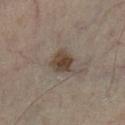* follow-up: no biopsy performed (imaged during a skin exam)
* image: total-body-photography crop, ~15 mm field of view
* location: the left lower leg
* lighting: cross-polarized illumination
* diameter: ≈3 mm
* patient: male, aged around 55